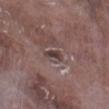Context: The patient is a male about 75 years old. A roughly 15 mm field-of-view crop from a total-body skin photograph. The lesion-visualizer software estimated a border-irregularity rating of about 3/10 and peripheral color asymmetry of about 0.5. Measured at roughly 2.5 mm in maximum diameter. This is a white-light tile. The lesion is located on the right lower leg.